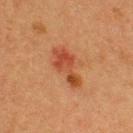Impression:
Part of a total-body skin-imaging series; this lesion was reviewed on a skin check and was not flagged for biopsy.
Image and clinical context:
A male subject aged approximately 40. The total-body-photography lesion software estimated a mean CIELAB color near L≈39 a*≈26 b*≈32, roughly 9 lightness units darker than nearby skin, and a normalized lesion–skin contrast near 7.5. Approximately 5 mm at its widest. A roughly 15 mm field-of-view crop from a total-body skin photograph. The lesion is on the upper back. This is a cross-polarized tile.